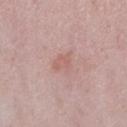Captured during whole-body skin photography for melanoma surveillance; the lesion was not biopsied. The tile uses white-light illumination. The lesion is located on the right lower leg. Approximately 3 mm at its widest. A female subject aged around 40. A region of skin cropped from a whole-body photographic capture, roughly 15 mm wide.